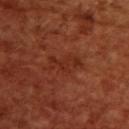Assessment: This lesion was catalogued during total-body skin photography and was not selected for biopsy. Acquisition and patient details: Located on the upper back. The patient is a female approximately 55 years of age. A 15 mm crop from a total-body photograph taken for skin-cancer surveillance.